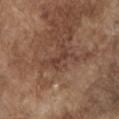This lesion was catalogued during total-body skin photography and was not selected for biopsy. The patient is a male aged 73 to 77. From the chest. This image is a 15 mm lesion crop taken from a total-body photograph. The tile uses white-light illumination. About 2.5 mm across.